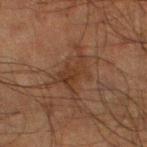Assessment:
This lesion was catalogued during total-body skin photography and was not selected for biopsy.
Image and clinical context:
This is a cross-polarized tile. Automated image analysis of the tile measured a border-irregularity rating of about 8/10 and peripheral color asymmetry of about 1. The analysis additionally found a nevus-likeness score of about 0/100 and lesion-presence confidence of about 85/100. A lesion tile, about 15 mm wide, cut from a 3D total-body photograph. On the left lower leg. The subject is a male aged around 50. Approximately 5.5 mm at its widest.